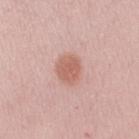The lesion was photographed on a routine skin check and not biopsied; there is no pathology result.
A female patient roughly 30 years of age.
The lesion is on the right upper arm.
The lesion's longest dimension is about 3.5 mm.
The tile uses white-light illumination.
An algorithmic analysis of the crop reported a footprint of about 8 mm², an eccentricity of roughly 0.6, and two-axis asymmetry of about 0.2.
A region of skin cropped from a whole-body photographic capture, roughly 15 mm wide.